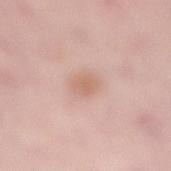Imaged during a routine full-body skin examination; the lesion was not biopsied and no histopathology is available.
Captured under white-light illumination.
The patient is a female approximately 50 years of age.
A 15 mm crop from a total-body photograph taken for skin-cancer surveillance.
Longest diameter approximately 2.5 mm.
From the right lower leg.